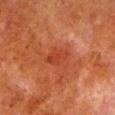Impression: Part of a total-body skin-imaging series; this lesion was reviewed on a skin check and was not flagged for biopsy. Acquisition and patient details: A male subject, aged around 80. A 15 mm close-up tile from a total-body photography series done for melanoma screening. Longest diameter approximately 3 mm. Captured under cross-polarized illumination. The lesion is on the left lower leg.